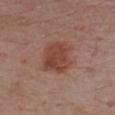biopsy status = no biopsy performed (imaged during a skin exam)
image source = ~15 mm crop, total-body skin-cancer survey
site = the chest
automated metrics = a border-irregularity index near 2/10, a within-lesion color-variation index near 4/10, and peripheral color asymmetry of about 1.5; a classifier nevus-likeness of about 95/100 and a detector confidence of about 100 out of 100 that the crop contains a lesion
patient = male, about 75 years old
lighting = white-light